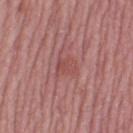Impression: The lesion was photographed on a routine skin check and not biopsied; there is no pathology result. Clinical summary: Cropped from a total-body skin-imaging series; the visible field is about 15 mm. A female subject, about 50 years old. From the left thigh.